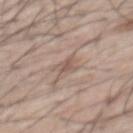Clinical impression: The lesion was tiled from a total-body skin photograph and was not biopsied. Background: A 15 mm crop from a total-body photograph taken for skin-cancer surveillance. A male patient, in their mid-50s. The total-body-photography lesion software estimated an average lesion color of about L≈56 a*≈16 b*≈24 (CIELAB), a lesion–skin lightness drop of about 8, and a normalized lesion–skin contrast near 5.5. It also reported border irregularity of about 4 on a 0–10 scale. Located on the mid back. Approximately 3 mm at its widest. The tile uses white-light illumination.